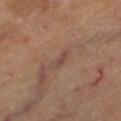Notes:
* biopsy status: imaged on a skin check; not biopsied
* location: the left thigh
* image source: 15 mm crop, total-body photography
* subject: in their 60s
* lesion size: about 2.5 mm